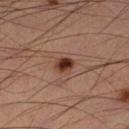Q: Was this lesion biopsied?
A: catalogued during a skin exam; not biopsied
Q: Illumination type?
A: cross-polarized
Q: What did automated image analysis measure?
A: a lesion area of about 3.5 mm², an eccentricity of roughly 0.55, and two-axis asymmetry of about 0.25; a classifier nevus-likeness of about 100/100 and lesion-presence confidence of about 100/100
Q: What is the lesion's diameter?
A: about 2 mm
Q: How was this image acquired?
A: ~15 mm crop, total-body skin-cancer survey
Q: Who is the patient?
A: male, aged around 45
Q: Where on the body is the lesion?
A: the left lower leg